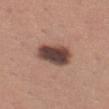- size · ≈4.5 mm
- anatomic site · the left thigh
- patient · female, in their 30s
- image · ~15 mm tile from a whole-body skin photo